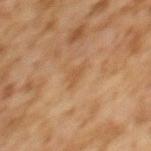No biopsy was performed on this lesion — it was imaged during a full skin examination and was not determined to be concerning. A 15 mm crop from a total-body photograph taken for skin-cancer surveillance. The lesion is on the back. A female patient, aged 58–62.